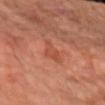illumination = cross-polarized illumination; acquisition = 15 mm crop, total-body photography; site = the left forearm; lesion diameter = ≈3.5 mm; patient = female, aged around 65.